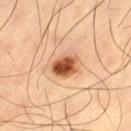No biopsy was performed on this lesion — it was imaged during a full skin examination and was not determined to be concerning. A male subject aged 58 to 62. A 15 mm crop from a total-body photograph taken for skin-cancer surveillance. The recorded lesion diameter is about 3.5 mm. From the left thigh.